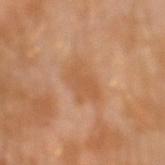Q: Was a biopsy performed?
A: imaged on a skin check; not biopsied
Q: How was the tile lit?
A: cross-polarized
Q: What is the anatomic site?
A: the left forearm
Q: How was this image acquired?
A: ~15 mm crop, total-body skin-cancer survey
Q: Patient demographics?
A: male, aged approximately 30